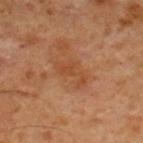• follow-up · no biopsy performed (imaged during a skin exam)
• subject · male, about 60 years old
• body site · the right lower leg
• TBP lesion metrics · an area of roughly 6 mm², an eccentricity of roughly 0.85, and a shape-asymmetry score of about 0.5 (0 = symmetric); internal color variation of about 1.5 on a 0–10 scale and a peripheral color-asymmetry measure near 0.5
• lighting · cross-polarized
• lesion size · ≈4 mm
• imaging modality · total-body-photography crop, ~15 mm field of view